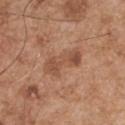No biopsy was performed on this lesion — it was imaged during a full skin examination and was not determined to be concerning. A roughly 15 mm field-of-view crop from a total-body skin photograph. The lesion is on the right upper arm. A male patient approximately 55 years of age.